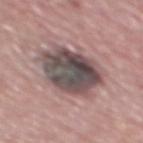Imaged during a routine full-body skin examination; the lesion was not biopsied and no histopathology is available. The lesion is located on the mid back. Captured under white-light illumination. A male subject, aged around 70. A 15 mm close-up extracted from a 3D total-body photography capture.